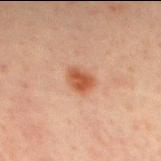{"biopsy_status": "not biopsied; imaged during a skin examination", "image": {"source": "total-body photography crop", "field_of_view_mm": 15}, "lesion_size": {"long_diameter_mm_approx": 3.0}, "automated_metrics": {"lesion_detection_confidence_0_100": 100}, "patient": {"sex": "male", "age_approx": 30}, "site": "mid back", "lighting": "cross-polarized"}